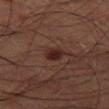Q: Was a biopsy performed?
A: no biopsy performed (imaged during a skin exam)
Q: Who is the patient?
A: male, approximately 60 years of age
Q: What kind of image is this?
A: total-body-photography crop, ~15 mm field of view
Q: How large is the lesion?
A: ≈2.5 mm
Q: What is the anatomic site?
A: the leg
Q: Automated lesion metrics?
A: a footprint of about 4 mm², an eccentricity of roughly 0.3, and two-axis asymmetry of about 0.2; a border-irregularity index near 1.5/10 and a peripheral color-asymmetry measure near 1